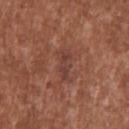Imaged during a routine full-body skin examination; the lesion was not biopsied and no histopathology is available.
An algorithmic analysis of the crop reported a lesion color around L≈41 a*≈23 b*≈25 in CIELAB. The analysis additionally found an automated nevus-likeness rating near 0 out of 100.
A male subject, aged 43–47.
The lesion is on the upper back.
A 15 mm close-up tile from a total-body photography series done for melanoma screening.
Approximately 4 mm at its widest.